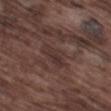Q: Is there a histopathology result?
A: imaged on a skin check; not biopsied
Q: Who is the patient?
A: male, aged around 75
Q: How was this image acquired?
A: ~15 mm crop, total-body skin-cancer survey
Q: What is the anatomic site?
A: the left thigh
Q: Automated lesion metrics?
A: a detector confidence of about 60 out of 100 that the crop contains a lesion